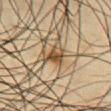notes: no biopsy performed (imaged during a skin exam)
lighting: cross-polarized
acquisition: ~15 mm tile from a whole-body skin photo
subject: male, about 35 years old
anatomic site: the chest